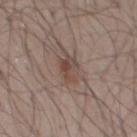Q: How was this image acquired?
A: ~15 mm crop, total-body skin-cancer survey
Q: Lesion location?
A: the mid back
Q: What is the lesion's diameter?
A: ≈4 mm
Q: Who is the patient?
A: male, in their 50s